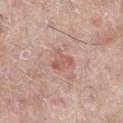<lesion>
<biopsy_status>not biopsied; imaged during a skin examination</biopsy_status>
<lighting>white-light</lighting>
<lesion_size>
  <long_diameter_mm_approx>2.5</long_diameter_mm_approx>
</lesion_size>
<automated_metrics>
  <area_mm2_approx>4.5</area_mm2_approx>
  <shape_asymmetry>0.5</shape_asymmetry>
  <cielab_L>57</cielab_L>
  <cielab_a>24</cielab_a>
  <cielab_b>25</cielab_b>
  <vs_skin_darker_L>8.0</vs_skin_darker_L>
  <vs_skin_contrast_norm>6.0</vs_skin_contrast_norm>
</automated_metrics>
<image>
  <source>total-body photography crop</source>
  <field_of_view_mm>15</field_of_view_mm>
</image>
<patient>
  <sex>female</sex>
  <age_approx>70</age_approx>
</patient>
<site>left lower leg</site>
</lesion>